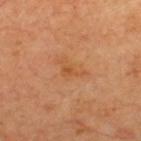The lesion was tiled from a total-body skin photograph and was not biopsied. Cropped from a whole-body photographic skin survey; the tile spans about 15 mm. The subject is in their mid-60s. From the upper back. Automated tile analysis of the lesion measured a detector confidence of about 100 out of 100 that the crop contains a lesion.